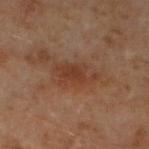Impression:
Imaged during a routine full-body skin examination; the lesion was not biopsied and no histopathology is available.
Clinical summary:
This is a cross-polarized tile. A male subject, about 60 years old. A region of skin cropped from a whole-body photographic capture, roughly 15 mm wide. The lesion is on the left lower leg.